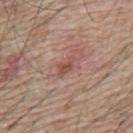follow-up=catalogued during a skin exam; not biopsied | site=the back | patient=male, aged 63–67 | image=total-body-photography crop, ~15 mm field of view.